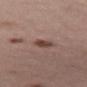Impression:
The lesion was photographed on a routine skin check and not biopsied; there is no pathology result.
Clinical summary:
A roughly 15 mm field-of-view crop from a total-body skin photograph. Approximately 2.5 mm at its widest. The lesion is located on the left thigh. The patient is a male aged approximately 65. This is a white-light tile. An algorithmic analysis of the crop reported a mean CIELAB color near L≈44 a*≈18 b*≈23, about 11 CIELAB-L* units darker than the surrounding skin, and a normalized lesion–skin contrast near 8.5.